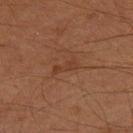Captured during whole-body skin photography for melanoma surveillance; the lesion was not biopsied.
Automated tile analysis of the lesion measured a mean CIELAB color near L≈31 a*≈19 b*≈26, roughly 5 lightness units darker than nearby skin, and a lesion-to-skin contrast of about 5 (normalized; higher = more distinct). The analysis additionally found a border-irregularity index near 5.5/10, a color-variation rating of about 0/10, and a peripheral color-asymmetry measure near 0. It also reported a nevus-likeness score of about 0/100 and a detector confidence of about 100 out of 100 that the crop contains a lesion.
Imaged with cross-polarized lighting.
A male subject aged around 60.
On the right thigh.
A 15 mm close-up extracted from a 3D total-body photography capture.